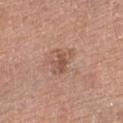Findings:
• notes — total-body-photography surveillance lesion; no biopsy
• image source — 15 mm crop, total-body photography
• lighting — white-light
• location — the leg
• patient — female, aged 73–77
• automated lesion analysis — an eccentricity of roughly 0.8; a lesion color around L≈53 a*≈21 b*≈29 in CIELAB, about 9 CIELAB-L* units darker than the surrounding skin, and a normalized lesion–skin contrast near 6; a border-irregularity rating of about 4.5/10, a within-lesion color-variation index near 2/10, and radial color variation of about 0.5
• lesion size — ~3.5 mm (longest diameter)